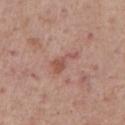Impression: Captured during whole-body skin photography for melanoma surveillance; the lesion was not biopsied. Clinical summary: A male subject about 75 years old. Cropped from a whole-body photographic skin survey; the tile spans about 15 mm. Automated tile analysis of the lesion measured an area of roughly 4.5 mm², an eccentricity of roughly 0.9, and a shape-asymmetry score of about 0.55 (0 = symmetric). The analysis additionally found a lesion color around L≈53 a*≈23 b*≈26 in CIELAB and a lesion–skin lightness drop of about 8. And it measured a border-irregularity index near 6/10, a color-variation rating of about 1.5/10, and peripheral color asymmetry of about 0.5. It also reported an automated nevus-likeness rating near 5 out of 100 and a detector confidence of about 100 out of 100 that the crop contains a lesion. The tile uses white-light illumination. The lesion is located on the chest.